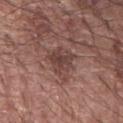{
  "biopsy_status": "not biopsied; imaged during a skin examination",
  "patient": {
    "sex": "male",
    "age_approx": 70
  },
  "lighting": "white-light",
  "site": "right forearm",
  "image": {
    "source": "total-body photography crop",
    "field_of_view_mm": 15
  },
  "automated_metrics": {
    "cielab_L": 42,
    "cielab_a": 20,
    "cielab_b": 22,
    "vs_skin_darker_L": 9.0,
    "vs_skin_contrast_norm": 7.0,
    "border_irregularity_0_10": 4.5,
    "color_variation_0_10": 3.5,
    "peripheral_color_asymmetry": 1.5,
    "nevus_likeness_0_100": 25,
    "lesion_detection_confidence_0_100": 100
  },
  "lesion_size": {
    "long_diameter_mm_approx": 4.0
  }
}